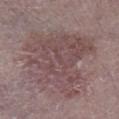Clinical impression: The lesion was tiled from a total-body skin photograph and was not biopsied. Clinical summary: The lesion is on the right lower leg. The patient is a male aged approximately 75. A 15 mm crop from a total-body photograph taken for skin-cancer surveillance.